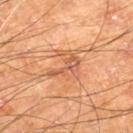Part of a total-body skin-imaging series; this lesion was reviewed on a skin check and was not flagged for biopsy.
Longest diameter approximately 4 mm.
The lesion-visualizer software estimated a color-variation rating of about 6.5/10 and radial color variation of about 2.
On the right lower leg.
A male patient, aged approximately 70.
A 15 mm close-up extracted from a 3D total-body photography capture.